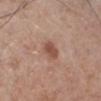{"biopsy_status": "not biopsied; imaged during a skin examination", "patient": {"sex": "male", "age_approx": 70}, "lighting": "white-light", "image": {"source": "total-body photography crop", "field_of_view_mm": 15}, "site": "right lower leg", "lesion_size": {"long_diameter_mm_approx": 3.0}}